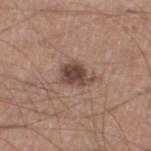biopsy status — catalogued during a skin exam; not biopsied | automated metrics — a mean CIELAB color near L≈44 a*≈18 b*≈23, a lesion–skin lightness drop of about 13, and a lesion-to-skin contrast of about 10 (normalized; higher = more distinct); a border-irregularity rating of about 3.5/10 and a peripheral color-asymmetry measure near 1.5; lesion-presence confidence of about 100/100 | illumination — white-light illumination | body site — the left lower leg | subject — male, aged 18–22 | image source — 15 mm crop, total-body photography.